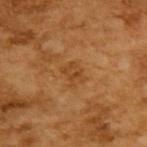biopsy_status: not biopsied; imaged during a skin examination
automated_metrics:
  area_mm2_approx: 4.0
  border_irregularity_0_10: 3.5
  color_variation_0_10: 3.0
  peripheral_color_asymmetry: 1.0
  nevus_likeness_0_100: 0
  lesion_detection_confidence_0_100: 100
patient:
  sex: male
  age_approx: 65
lesion_size:
  long_diameter_mm_approx: 2.5
image:
  source: total-body photography crop
  field_of_view_mm: 15
lighting: cross-polarized